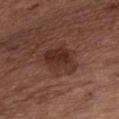Notes:
- notes: imaged on a skin check; not biopsied
- subject: male, aged 48–52
- image: ~15 mm crop, total-body skin-cancer survey
- diameter: about 4.5 mm
- anatomic site: the front of the torso